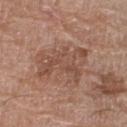No biopsy was performed on this lesion — it was imaged during a full skin examination and was not determined to be concerning. About 6 mm across. Cropped from a total-body skin-imaging series; the visible field is about 15 mm. The lesion is on the leg. Imaged with white-light lighting. A female subject, aged 78 to 82.